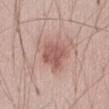  biopsy_status: not biopsied; imaged during a skin examination
  automated_metrics:
    border_irregularity_0_10: 2.5
    color_variation_0_10: 2.5
    peripheral_color_asymmetry: 1.0
    nevus_likeness_0_100: 20
    lesion_detection_confidence_0_100: 100
  site: abdomen
  image:
    source: total-body photography crop
    field_of_view_mm: 15
  patient:
    sex: male
    age_approx: 45
  lesion_size:
    long_diameter_mm_approx: 3.5